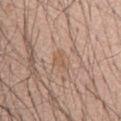follow-up: total-body-photography surveillance lesion; no biopsy | image source: 15 mm crop, total-body photography | patient: male, aged 58 to 62 | body site: the mid back.